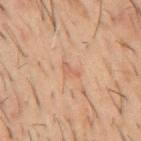Part of a total-body skin-imaging series; this lesion was reviewed on a skin check and was not flagged for biopsy. The lesion's longest dimension is about 2.5 mm. Cropped from a total-body skin-imaging series; the visible field is about 15 mm. A male subject roughly 60 years of age. On the mid back. This is a cross-polarized tile.